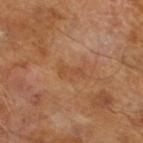biopsy status = catalogued during a skin exam; not biopsied
imaging modality = ~15 mm tile from a whole-body skin photo
lesion size = about 3 mm
lighting = cross-polarized
subject = male, aged approximately 65